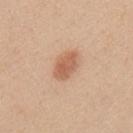Imaged during a routine full-body skin examination; the lesion was not biopsied and no histopathology is available. A male subject aged 33–37. The lesion is on the upper back. Cropped from a whole-body photographic skin survey; the tile spans about 15 mm. The tile uses white-light illumination. About 4 mm across.